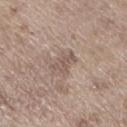A 15 mm crop from a total-body photograph taken for skin-cancer surveillance. On the left lower leg. Imaged with white-light lighting. A male subject, aged approximately 70.